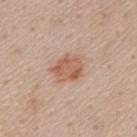Clinical impression:
The lesion was photographed on a routine skin check and not biopsied; there is no pathology result.
Image and clinical context:
Cropped from a total-body skin-imaging series; the visible field is about 15 mm. The subject is a male aged approximately 50. Measured at roughly 3.5 mm in maximum diameter. The lesion is located on the back.